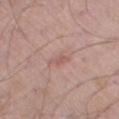Clinical impression:
Recorded during total-body skin imaging; not selected for excision or biopsy.
Image and clinical context:
The lesion is on the leg. A 15 mm close-up tile from a total-body photography series done for melanoma screening. The recorded lesion diameter is about 2.5 mm. A male patient, aged 68 to 72.